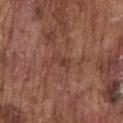| key | value |
|---|---|
| follow-up | total-body-photography surveillance lesion; no biopsy |
| automated lesion analysis | a shape-asymmetry score of about 0.45 (0 = symmetric); a lesion color around L≈39 a*≈22 b*≈26 in CIELAB, a lesion–skin lightness drop of about 6, and a normalized border contrast of about 5.5; a border-irregularity rating of about 4.5/10 and radial color variation of about 0 |
| lesion diameter | ≈2.5 mm |
| image source | ~15 mm tile from a whole-body skin photo |
| tile lighting | white-light |
| subject | male, aged 73 to 77 |
| location | the chest |